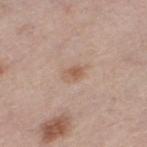Q: Was this lesion biopsied?
A: no biopsy performed (imaged during a skin exam)
Q: Lesion location?
A: the left thigh
Q: Who is the patient?
A: female, aged around 65
Q: How was this image acquired?
A: ~15 mm tile from a whole-body skin photo